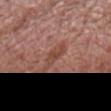workup = catalogued during a skin exam; not biopsied
acquisition = total-body-photography crop, ~15 mm field of view
location = the head or neck
patient = male, roughly 70 years of age